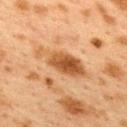Impression:
Captured during whole-body skin photography for melanoma surveillance; the lesion was not biopsied.
Clinical summary:
An algorithmic analysis of the crop reported a lesion area of about 13 mm² and a symmetry-axis asymmetry near 0.25. And it measured an average lesion color of about L≈46 a*≈20 b*≈35 (CIELAB), a lesion–skin lightness drop of about 11, and a lesion-to-skin contrast of about 9 (normalized; higher = more distinct). The analysis additionally found a border-irregularity rating of about 4/10, a within-lesion color-variation index near 5.5/10, and a peripheral color-asymmetry measure near 2. A region of skin cropped from a whole-body photographic capture, roughly 15 mm wide. The lesion is on the upper back. Imaged with cross-polarized lighting. About 7 mm across. The patient is a female aged around 40.